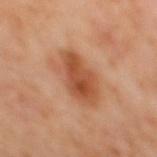<lesion>
  <biopsy_status>not biopsied; imaged during a skin examination</biopsy_status>
  <image>
    <source>total-body photography crop</source>
    <field_of_view_mm>15</field_of_view_mm>
  </image>
  <lesion_size>
    <long_diameter_mm_approx>5.5</long_diameter_mm_approx>
  </lesion_size>
  <patient>
    <sex>male</sex>
    <age_approx>60</age_approx>
  </patient>
  <site>mid back</site>
</lesion>